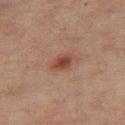Notes:
• lesion diameter · about 2.5 mm
• illumination · cross-polarized illumination
• subject · female, aged approximately 55
• acquisition · ~15 mm crop, total-body skin-cancer survey
• body site · the leg